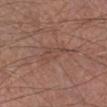notes=catalogued during a skin exam; not biopsied
image=15 mm crop, total-body photography
patient=male, about 55 years old
site=the right lower leg
lesion size=≈5.5 mm